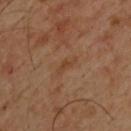{
  "biopsy_status": "not biopsied; imaged during a skin examination",
  "site": "upper back",
  "patient": {
    "sex": "male",
    "age_approx": 45
  },
  "image": {
    "source": "total-body photography crop",
    "field_of_view_mm": 15
  }
}